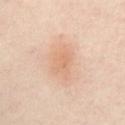Assessment: The lesion was tiled from a total-body skin photograph and was not biopsied. Context: The subject is a female aged 53–57. Approximately 3.5 mm at its widest. The lesion is on the abdomen. A 15 mm close-up tile from a total-body photography series done for melanoma screening. The tile uses cross-polarized illumination.